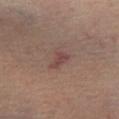Recorded during total-body skin imaging; not selected for excision or biopsy. The subject is a male in their mid-40s. The total-body-photography lesion software estimated an area of roughly 3.5 mm², an eccentricity of roughly 0.8, and a symmetry-axis asymmetry near 0.4. The software also gave an average lesion color of about L≈40 a*≈19 b*≈19 (CIELAB), a lesion–skin lightness drop of about 7, and a normalized border contrast of about 6.5. The software also gave a detector confidence of about 100 out of 100 that the crop contains a lesion. A 15 mm crop from a total-body photograph taken for skin-cancer surveillance. The lesion is on the left lower leg. Approximately 2.5 mm at its widest.